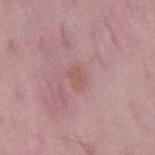Impression: Imaged during a routine full-body skin examination; the lesion was not biopsied and no histopathology is available. Clinical summary: The lesion is on the mid back. A male subject, aged 48 to 52. This image is a 15 mm lesion crop taken from a total-body photograph.